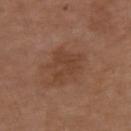This is a white-light tile.
The subject is a male in their mid- to late 70s.
A 15 mm close-up extracted from a 3D total-body photography capture.
On the left upper arm.
Automated tile analysis of the lesion measured a footprint of about 9 mm² and a shape-asymmetry score of about 0.4 (0 = symmetric). And it measured a mean CIELAB color near L≈41 a*≈20 b*≈29, roughly 6 lightness units darker than nearby skin, and a normalized border contrast of about 5.5. The software also gave border irregularity of about 4.5 on a 0–10 scale, a color-variation rating of about 2.5/10, and radial color variation of about 1.